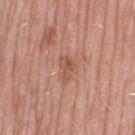Assessment:
This lesion was catalogued during total-body skin photography and was not selected for biopsy.
Background:
The subject is a female aged 68–72. On the left thigh. Cropped from a whole-body photographic skin survey; the tile spans about 15 mm.